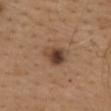The lesion was photographed on a routine skin check and not biopsied; there is no pathology result.
A 15 mm close-up tile from a total-body photography series done for melanoma screening.
Located on the upper back.
The total-body-photography lesion software estimated a lesion color around L≈43 a*≈18 b*≈29 in CIELAB and a lesion–skin lightness drop of about 12.
The patient is a female roughly 40 years of age.